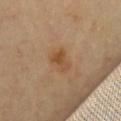No biopsy was performed on this lesion — it was imaged during a full skin examination and was not determined to be concerning.
Longest diameter approximately 3 mm.
A female patient, about 60 years old.
Located on the front of the torso.
The lesion-visualizer software estimated border irregularity of about 4.5 on a 0–10 scale and radial color variation of about 1.
Imaged with cross-polarized lighting.
A region of skin cropped from a whole-body photographic capture, roughly 15 mm wide.